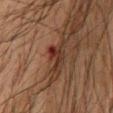workup: catalogued during a skin exam; not biopsied | lesion diameter: ≈3 mm | anatomic site: the chest | image-analysis metrics: an average lesion color of about L≈26 a*≈20 b*≈23 (CIELAB), a lesion–skin lightness drop of about 9, and a lesion-to-skin contrast of about 9.5 (normalized; higher = more distinct); border irregularity of about 7.5 on a 0–10 scale, a within-lesion color-variation index near 8.5/10, and a peripheral color-asymmetry measure near 3; a detector confidence of about 100 out of 100 that the crop contains a lesion | imaging modality: total-body-photography crop, ~15 mm field of view | subject: male, about 65 years old | tile lighting: cross-polarized.